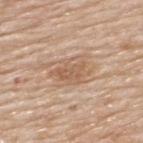Captured during whole-body skin photography for melanoma surveillance; the lesion was not biopsied.